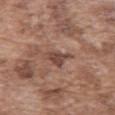workup=no biopsy performed (imaged during a skin exam); location=the front of the torso; tile lighting=white-light illumination; patient=male, approximately 75 years of age; diameter=≈4 mm; imaging modality=~15 mm crop, total-body skin-cancer survey.